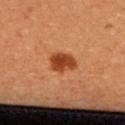Captured during whole-body skin photography for melanoma surveillance; the lesion was not biopsied.
Automated image analysis of the tile measured an area of roughly 7 mm², an eccentricity of roughly 0.7, and a shape-asymmetry score of about 0.25 (0 = symmetric). And it measured border irregularity of about 2 on a 0–10 scale, a within-lesion color-variation index near 3.5/10, and peripheral color asymmetry of about 1. And it measured a classifier nevus-likeness of about 100/100 and a detector confidence of about 100 out of 100 that the crop contains a lesion.
The lesion's longest dimension is about 3.5 mm.
The patient is a female aged around 50.
Captured under cross-polarized illumination.
This image is a 15 mm lesion crop taken from a total-body photograph.